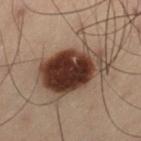Findings:
• biopsy status: no biopsy performed (imaged during a skin exam)
• site: the left thigh
• automated lesion analysis: an eccentricity of roughly 0.7 and two-axis asymmetry of about 0.2; an average lesion color of about L≈28 a*≈15 b*≈20 (CIELAB), about 16 CIELAB-L* units darker than the surrounding skin, and a lesion-to-skin contrast of about 15 (normalized; higher = more distinct)
• acquisition: 15 mm crop, total-body photography
• diameter: ~7.5 mm (longest diameter)
• patient: male, aged 53–57